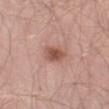follow-up=no biopsy performed (imaged during a skin exam)
location=the leg
illumination=white-light
acquisition=total-body-photography crop, ~15 mm field of view
patient=male, aged 53 to 57
automated lesion analysis=border irregularity of about 1.5 on a 0–10 scale, internal color variation of about 3.5 on a 0–10 scale, and radial color variation of about 1; a nevus-likeness score of about 90/100
lesion diameter=≈3 mm